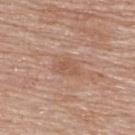{"biopsy_status": "not biopsied; imaged during a skin examination", "patient": {"sex": "female", "age_approx": 65}, "lighting": "white-light", "site": "upper back", "lesion_size": {"long_diameter_mm_approx": 3.5}, "image": {"source": "total-body photography crop", "field_of_view_mm": 15}}